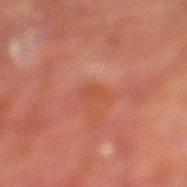Findings:
* subject — male, aged 68–72
* acquisition — total-body-photography crop, ~15 mm field of view
* body site — the left lower leg
* automated metrics — a lesion area of about 2.5 mm² and an eccentricity of roughly 0.9; a mean CIELAB color near L≈41 a*≈25 b*≈29 and a lesion–skin lightness drop of about 5; a classifier nevus-likeness of about 0/100 and a lesion-detection confidence of about 90/100
* diameter — ~2.5 mm (longest diameter)